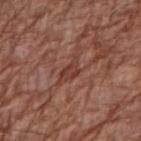image source — ~15 mm tile from a whole-body skin photo; patient — male, aged approximately 70; body site — the right upper arm.